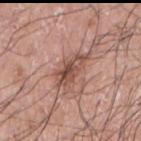Findings:
* workup — imaged on a skin check; not biopsied
* diameter — about 5 mm
* imaging modality — total-body-photography crop, ~15 mm field of view
* automated metrics — an area of roughly 9 mm² and a shape-asymmetry score of about 0.45 (0 = symmetric); an average lesion color of about L≈51 a*≈21 b*≈26 (CIELAB) and a normalized lesion–skin contrast near 8
* location — the left arm
* patient — male, aged 68–72